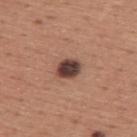notes: imaged on a skin check; not biopsied | imaging modality: ~15 mm crop, total-body skin-cancer survey | patient: male, aged 43–47 | body site: the back | lighting: white-light illumination | image-analysis metrics: an eccentricity of roughly 0.65 and a symmetry-axis asymmetry near 0.15; a mean CIELAB color near L≈40 a*≈20 b*≈22, about 18 CIELAB-L* units darker than the surrounding skin, and a lesion-to-skin contrast of about 14 (normalized; higher = more distinct); border irregularity of about 1.5 on a 0–10 scale, internal color variation of about 4 on a 0–10 scale, and radial color variation of about 1; an automated nevus-likeness rating near 50 out of 100 and a lesion-detection confidence of about 100/100.